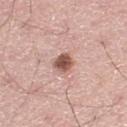Assessment: Part of a total-body skin-imaging series; this lesion was reviewed on a skin check and was not flagged for biopsy. Acquisition and patient details: From the left thigh. About 2.5 mm across. Cropped from a whole-body photographic skin survey; the tile spans about 15 mm. A male patient, aged around 60. The total-body-photography lesion software estimated a peripheral color-asymmetry measure near 1.